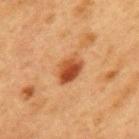Background:
This is a cross-polarized tile. A female patient aged approximately 40. Automated tile analysis of the lesion measured a lesion area of about 7.5 mm², an outline eccentricity of about 0.75 (0 = round, 1 = elongated), and two-axis asymmetry of about 0.2. And it measured a border-irregularity index near 2/10 and peripheral color asymmetry of about 2.5. And it measured a classifier nevus-likeness of about 100/100. A region of skin cropped from a whole-body photographic capture, roughly 15 mm wide. The lesion is on the mid back. Longest diameter approximately 4 mm.